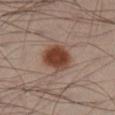No biopsy was performed on this lesion — it was imaged during a full skin examination and was not determined to be concerning. The subject is a male about 40 years old. A 15 mm close-up extracted from a 3D total-body photography capture. The lesion is located on the leg. An algorithmic analysis of the crop reported a peripheral color-asymmetry measure near 1. And it measured an automated nevus-likeness rating near 100 out of 100. About 4 mm across.